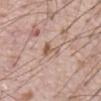<lesion>
<image>
  <source>total-body photography crop</source>
  <field_of_view_mm>15</field_of_view_mm>
</image>
<patient>
  <sex>male</sex>
  <age_approx>70</age_approx>
</patient>
<site>abdomen</site>
</lesion>